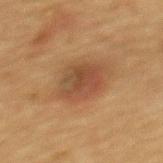notes: total-body-photography surveillance lesion; no biopsy | lesion diameter: ≈4.5 mm | image: 15 mm crop, total-body photography | anatomic site: the upper back | subject: female, aged approximately 70 | tile lighting: cross-polarized illumination.